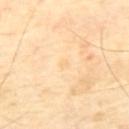| feature | finding |
|---|---|
| follow-up | no biopsy performed (imaged during a skin exam) |
| lesion diameter | about 1.5 mm |
| patient | male, in their mid- to late 60s |
| TBP lesion metrics | a footprint of about 1 mm², an eccentricity of roughly 0.7, and a symmetry-axis asymmetry near 0.4; a lesion-detection confidence of about 100/100 |
| site | the mid back |
| image source | total-body-photography crop, ~15 mm field of view |